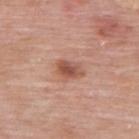The patient is a male aged 53 to 57. A 15 mm close-up extracted from a 3D total-body photography capture. The lesion is on the upper back. Imaged with white-light lighting.